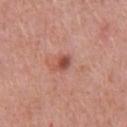<tbp_lesion>
<lighting>white-light</lighting>
<site>chest</site>
<patient>
  <sex>male</sex>
  <age_approx>50</age_approx>
</patient>
<image>
  <source>total-body photography crop</source>
  <field_of_view_mm>15</field_of_view_mm>
</image>
</tbp_lesion>